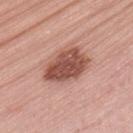Assessment:
This lesion was catalogued during total-body skin photography and was not selected for biopsy.
Clinical summary:
A female subject about 65 years old. The lesion is located on the left thigh. This image is a 15 mm lesion crop taken from a total-body photograph.